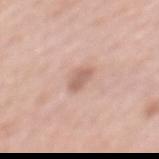image=total-body-photography crop, ~15 mm field of view
subject=female, in their mid-50s
anatomic site=the mid back
lesion size=about 3 mm
illumination=white-light